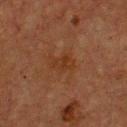Q: Is there a histopathology result?
A: catalogued during a skin exam; not biopsied
Q: What is the anatomic site?
A: the front of the torso
Q: Who is the patient?
A: male, approximately 75 years of age
Q: How was this image acquired?
A: 15 mm crop, total-body photography
Q: What is the lesion's diameter?
A: ≈3 mm
Q: What did automated image analysis measure?
A: a footprint of about 4 mm² and a shape-asymmetry score of about 0.25 (0 = symmetric); a classifier nevus-likeness of about 0/100 and a lesion-detection confidence of about 100/100
Q: What lighting was used for the tile?
A: cross-polarized illumination